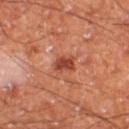Imaged during a routine full-body skin examination; the lesion was not biopsied and no histopathology is available. Imaged with cross-polarized lighting. Approximately 2.5 mm at its widest. A male subject, aged 58 to 62. Located on the right thigh. A region of skin cropped from a whole-body photographic capture, roughly 15 mm wide.